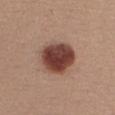{"biopsy_status": "not biopsied; imaged during a skin examination", "site": "right upper arm", "automated_metrics": {"cielab_L": 43, "cielab_a": 22, "cielab_b": 25, "vs_skin_darker_L": 18.0, "vs_skin_contrast_norm": 12.5}, "lighting": "white-light", "image": {"source": "total-body photography crop", "field_of_view_mm": 15}, "patient": {"sex": "female", "age_approx": 30}}